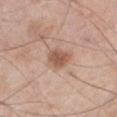Image and clinical context:
Imaged with white-light lighting. The total-body-photography lesion software estimated a lesion color around L≈55 a*≈21 b*≈29 in CIELAB, a lesion–skin lightness drop of about 11, and a normalized border contrast of about 8. And it measured a color-variation rating of about 2/10 and a peripheral color-asymmetry measure near 0.5. The analysis additionally found a nevus-likeness score of about 90/100. A roughly 15 mm field-of-view crop from a total-body skin photograph. A male patient roughly 70 years of age. The recorded lesion diameter is about 3 mm. Located on the abdomen.